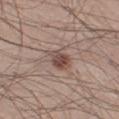Clinical impression:
No biopsy was performed on this lesion — it was imaged during a full skin examination and was not determined to be concerning.
Image and clinical context:
A male subject, in their mid- to late 20s. The total-body-photography lesion software estimated an area of roughly 5 mm², a shape eccentricity near 0.75, and a shape-asymmetry score of about 0.25 (0 = symmetric). Captured under white-light illumination. Approximately 3 mm at its widest. The lesion is on the right lower leg. A region of skin cropped from a whole-body photographic capture, roughly 15 mm wide.